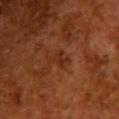follow-up = no biopsy performed (imaged during a skin exam) | imaging modality = ~15 mm crop, total-body skin-cancer survey | location = the upper back | illumination = cross-polarized | diameter = ~3 mm (longest diameter) | subject = female, aged around 50.